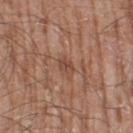Notes:
- workup — total-body-photography surveillance lesion; no biopsy
- acquisition — 15 mm crop, total-body photography
- lesion size — about 2.5 mm
- location — the right thigh
- TBP lesion metrics — a footprint of about 3.5 mm², an eccentricity of roughly 0.75, and two-axis asymmetry of about 0.25
- patient — male, in their 60s
- illumination — white-light